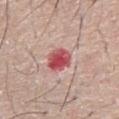Impression: Part of a total-body skin-imaging series; this lesion was reviewed on a skin check and was not flagged for biopsy. Context: Imaged with white-light lighting. The lesion is on the abdomen. Cropped from a whole-body photographic skin survey; the tile spans about 15 mm. Approximately 3.5 mm at its widest. An algorithmic analysis of the crop reported an average lesion color of about L≈53 a*≈36 b*≈23 (CIELAB), about 16 CIELAB-L* units darker than the surrounding skin, and a normalized border contrast of about 10.5. A male subject, aged 63–67.